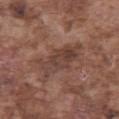Captured during whole-body skin photography for melanoma surveillance; the lesion was not biopsied. The lesion's longest dimension is about 6 mm. The tile uses white-light illumination. The lesion is on the abdomen. A male patient roughly 75 years of age. This image is a 15 mm lesion crop taken from a total-body photograph.